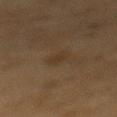follow-up: total-body-photography surveillance lesion; no biopsy
patient: male, aged around 85 or older
image: ~15 mm crop, total-body skin-cancer survey
location: the mid back